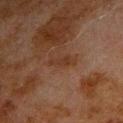workup — imaged on a skin check; not biopsied | tile lighting — cross-polarized illumination | body site — the left upper arm | image source — total-body-photography crop, ~15 mm field of view | automated metrics — a mean CIELAB color near L≈26 a*≈16 b*≈25 and a lesion–skin lightness drop of about 4; a border-irregularity index near 5/10; an automated nevus-likeness rating near 0 out of 100 and a lesion-detection confidence of about 100/100 | subject — male, in their 80s | size — about 2.5 mm.